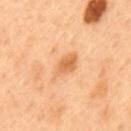Captured during whole-body skin photography for melanoma surveillance; the lesion was not biopsied. The subject is a male aged 48 to 52. A roughly 15 mm field-of-view crop from a total-body skin photograph. The lesion is located on the mid back. About 4.5 mm across.